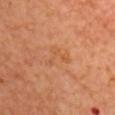Case summary:
- workup — no biopsy performed (imaged during a skin exam)